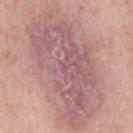Clinical impression: Recorded during total-body skin imaging; not selected for excision or biopsy. Background: A 15 mm close-up tile from a total-body photography series done for melanoma screening. A male patient roughly 65 years of age. The lesion-visualizer software estimated a lesion area of about 65 mm² and an eccentricity of roughly 0.9. The software also gave a mean CIELAB color near L≈58 a*≈22 b*≈19. The software also gave an automated nevus-likeness rating near 0 out of 100 and a lesion-detection confidence of about 95/100. On the chest. The lesion's longest dimension is about 14 mm.